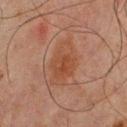Assessment: Part of a total-body skin-imaging series; this lesion was reviewed on a skin check and was not flagged for biopsy. Acquisition and patient details: Captured under cross-polarized illumination. A roughly 15 mm field-of-view crop from a total-body skin photograph. A male patient aged approximately 65. The lesion is on the upper back.